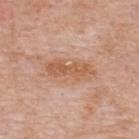Q: Was this lesion biopsied?
A: no biopsy performed (imaged during a skin exam)
Q: Where on the body is the lesion?
A: the upper back
Q: What kind of image is this?
A: 15 mm crop, total-body photography
Q: What did automated image analysis measure?
A: an area of roughly 9 mm², a shape eccentricity near 0.95, and a symmetry-axis asymmetry near 0.2; a border-irregularity index near 4/10 and a color-variation rating of about 2.5/10; a classifier nevus-likeness of about 0/100
Q: Patient demographics?
A: male, about 75 years old
Q: Illumination type?
A: white-light illumination